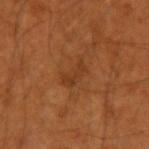Recorded during total-body skin imaging; not selected for excision or biopsy.
A male patient in their 50s.
Approximately 3.5 mm at its widest.
A 15 mm close-up extracted from a 3D total-body photography capture.
On the left upper arm.
The tile uses cross-polarized illumination.